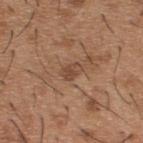image source — ~15 mm crop, total-body skin-cancer survey; lesion diameter — ≈2.5 mm; anatomic site — the upper back; patient — male, approximately 40 years of age; lighting — white-light.